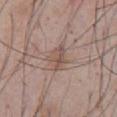biopsy status=total-body-photography surveillance lesion; no biopsy
automated lesion analysis=an area of roughly 5 mm², a shape eccentricity near 0.7, and two-axis asymmetry of about 0.35; a mean CIELAB color near L≈52 a*≈16 b*≈23 and a normalized lesion–skin contrast near 6.5
tile lighting=white-light illumination
subject=male, in their mid- to late 50s
acquisition=~15 mm tile from a whole-body skin photo
diameter=~3 mm (longest diameter)
body site=the abdomen